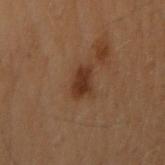Clinical impression:
The lesion was tiled from a total-body skin photograph and was not biopsied.
Image and clinical context:
A region of skin cropped from a whole-body photographic capture, roughly 15 mm wide. The subject is a female roughly 50 years of age. The lesion's longest dimension is about 3 mm. The tile uses cross-polarized illumination. The lesion is located on the arm.